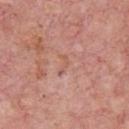Recorded during total-body skin imaging; not selected for excision or biopsy. Longest diameter approximately 2.5 mm. On the chest. A male subject, aged around 75. This is a white-light tile. A 15 mm crop from a total-body photograph taken for skin-cancer surveillance.